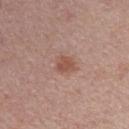No biopsy was performed on this lesion — it was imaged during a full skin examination and was not determined to be concerning. Imaged with white-light lighting. A female patient aged approximately 50. Longest diameter approximately 2.5 mm. The lesion is located on the arm. The lesion-visualizer software estimated an outline eccentricity of about 0.55 (0 = round, 1 = elongated) and two-axis asymmetry of about 0.2. It also reported an automated nevus-likeness rating near 70 out of 100 and lesion-presence confidence of about 100/100. A 15 mm close-up tile from a total-body photography series done for melanoma screening.